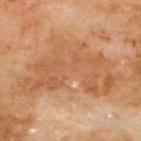{
  "biopsy_status": "not biopsied; imaged during a skin examination",
  "image": {
    "source": "total-body photography crop",
    "field_of_view_mm": 15
  },
  "site": "upper back",
  "lighting": "cross-polarized",
  "lesion_size": {
    "long_diameter_mm_approx": 11.5
  },
  "automated_metrics": {
    "nevus_likeness_0_100": 0
  },
  "patient": {
    "sex": "male",
    "age_approx": 70
  }
}